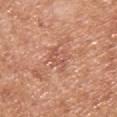Q: Where on the body is the lesion?
A: the chest
Q: How was the tile lit?
A: white-light
Q: How was this image acquired?
A: total-body-photography crop, ~15 mm field of view
Q: What are the patient's age and sex?
A: male, aged approximately 30
Q: How large is the lesion?
A: ≈3 mm
Q: Automated lesion metrics?
A: a mean CIELAB color near L≈56 a*≈25 b*≈31 and about 7 CIELAB-L* units darker than the surrounding skin; a border-irregularity index near 5/10, a color-variation rating of about 3/10, and radial color variation of about 1; a classifier nevus-likeness of about 0/100